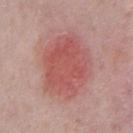<case>
  <biopsy_status>not biopsied; imaged during a skin examination</biopsy_status>
  <lighting>white-light</lighting>
  <patient>
    <sex>male</sex>
    <age_approx>40</age_approx>
  </patient>
  <site>chest</site>
  <image>
    <source>total-body photography crop</source>
    <field_of_view_mm>15</field_of_view_mm>
  </image>
  <lesion_size>
    <long_diameter_mm_approx>7.5</long_diameter_mm_approx>
  </lesion_size>
  <automated_metrics>
    <area_mm2_approx>34.0</area_mm2_approx>
    <eccentricity>0.55</eccentricity>
    <shape_asymmetry>0.15</shape_asymmetry>
  </automated_metrics>
</case>